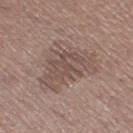follow-up: catalogued during a skin exam; not biopsied | image source: ~15 mm tile from a whole-body skin photo | anatomic site: the right thigh | patient: female, in their mid- to late 40s | automated lesion analysis: a footprint of about 24 mm², an outline eccentricity of about 0.5 (0 = round, 1 = elongated), and two-axis asymmetry of about 0.45; an average lesion color of about L≈50 a*≈15 b*≈22 (CIELAB) and a lesion-to-skin contrast of about 5.5 (normalized; higher = more distinct); an automated nevus-likeness rating near 0 out of 100 | lesion diameter: ≈6.5 mm | lighting: white-light illumination.